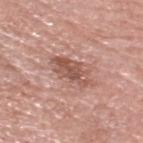follow-up=total-body-photography surveillance lesion; no biopsy | imaging modality=~15 mm tile from a whole-body skin photo | lesion diameter=about 5 mm | patient=male, approximately 70 years of age | lighting=white-light | location=the head or neck.